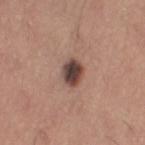Recorded during total-body skin imaging; not selected for excision or biopsy. Imaged with white-light lighting. A female subject approximately 65 years of age. A 15 mm close-up tile from a total-body photography series done for melanoma screening. Longest diameter approximately 3 mm. The lesion is on the leg. An algorithmic analysis of the crop reported a mean CIELAB color near L≈43 a*≈18 b*≈22, a lesion–skin lightness drop of about 17, and a lesion-to-skin contrast of about 12.5 (normalized; higher = more distinct). It also reported a border-irregularity index near 1/10, a color-variation rating of about 5/10, and radial color variation of about 1.5. The analysis additionally found a nevus-likeness score of about 35/100 and lesion-presence confidence of about 100/100.